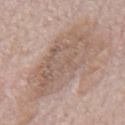Findings:
- follow-up: catalogued during a skin exam; not biopsied